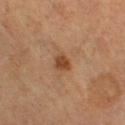This lesion was catalogued during total-body skin photography and was not selected for biopsy.
The subject is a female aged approximately 70.
Located on the right thigh.
Approximately 2.5 mm at its widest.
A 15 mm crop from a total-body photograph taken for skin-cancer surveillance.